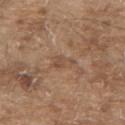Findings:
* notes · no biopsy performed (imaged during a skin exam)
* location · the upper back
* image · ~15 mm crop, total-body skin-cancer survey
* patient · male, aged approximately 80